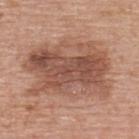| key | value |
|---|---|
| workup | total-body-photography surveillance lesion; no biopsy |
| location | the back |
| illumination | white-light |
| lesion diameter | about 9.5 mm |
| image | ~15 mm crop, total-body skin-cancer survey |
| subject | female, aged around 65 |